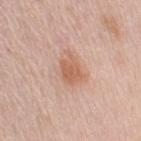Findings:
* biopsy status: no biopsy performed (imaged during a skin exam)
* image: 15 mm crop, total-body photography
* subject: female, aged approximately 45
* lesion diameter: about 4 mm
* anatomic site: the arm
* automated metrics: an eccentricity of roughly 0.75 and two-axis asymmetry of about 0.3; border irregularity of about 3 on a 0–10 scale and a color-variation rating of about 3.5/10; a classifier nevus-likeness of about 75/100 and lesion-presence confidence of about 100/100
* tile lighting: white-light